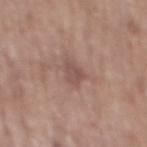The lesion was tiled from a total-body skin photograph and was not biopsied. A 15 mm crop from a total-body photograph taken for skin-cancer surveillance. The lesion-visualizer software estimated a lesion area of about 4.5 mm². The analysis additionally found an average lesion color of about L≈50 a*≈19 b*≈23 (CIELAB) and a lesion–skin lightness drop of about 8. The software also gave a border-irregularity index near 3.5/10, a within-lesion color-variation index near 2/10, and radial color variation of about 0.5. It also reported a nevus-likeness score of about 0/100 and lesion-presence confidence of about 100/100. The lesion is located on the back. The tile uses white-light illumination. Measured at roughly 3 mm in maximum diameter. A male subject aged 63 to 67.